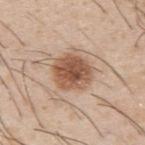No biopsy was performed on this lesion — it was imaged during a full skin examination and was not determined to be concerning.
A male subject aged 28–32.
The lesion is on the upper back.
Longest diameter approximately 4.5 mm.
Automated image analysis of the tile measured a footprint of about 15 mm², a shape eccentricity near 0.4, and two-axis asymmetry of about 0.15. And it measured a border-irregularity index near 1.5/10, a color-variation rating of about 5/10, and peripheral color asymmetry of about 1.5. The software also gave a nevus-likeness score of about 95/100 and a lesion-detection confidence of about 100/100.
This image is a 15 mm lesion crop taken from a total-body photograph.